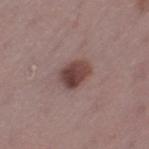Q: Was this lesion biopsied?
A: total-body-photography surveillance lesion; no biopsy
Q: Patient demographics?
A: female, in their mid- to late 40s
Q: Automated lesion metrics?
A: a border-irregularity rating of about 1.5/10, a within-lesion color-variation index near 5.5/10, and radial color variation of about 2; an automated nevus-likeness rating near 95 out of 100 and a lesion-detection confidence of about 100/100
Q: What is the imaging modality?
A: ~15 mm tile from a whole-body skin photo
Q: How was the tile lit?
A: white-light
Q: Lesion size?
A: ~3.5 mm (longest diameter)
Q: What is the anatomic site?
A: the left thigh